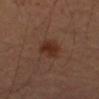Q: Was a biopsy performed?
A: total-body-photography surveillance lesion; no biopsy
Q: How was the tile lit?
A: cross-polarized illumination
Q: What are the patient's age and sex?
A: male, aged 63–67
Q: What is the lesion's diameter?
A: ~3 mm (longest diameter)
Q: What is the anatomic site?
A: the left forearm
Q: What did automated image analysis measure?
A: a lesion area of about 6.5 mm², a shape eccentricity near 0.35, and a shape-asymmetry score of about 0.2 (0 = symmetric); a lesion color around L≈29 a*≈20 b*≈26 in CIELAB, about 8 CIELAB-L* units darker than the surrounding skin, and a normalized border contrast of about 8; a border-irregularity rating of about 2/10, a within-lesion color-variation index near 2/10, and peripheral color asymmetry of about 0.5; a classifier nevus-likeness of about 95/100
Q: What kind of image is this?
A: ~15 mm tile from a whole-body skin photo